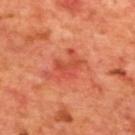Clinical impression: No biopsy was performed on this lesion — it was imaged during a full skin examination and was not determined to be concerning. Background: A male patient aged 68–72. Captured under cross-polarized illumination. The lesion is located on the mid back. This image is a 15 mm lesion crop taken from a total-body photograph.